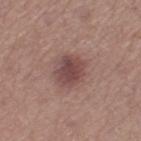biopsy status: catalogued during a skin exam; not biopsied
location: the left thigh
patient: female, in their mid-50s
image-analysis metrics: a lesion area of about 10 mm² and an outline eccentricity of about 0.6 (0 = round, 1 = elongated); a border-irregularity rating of about 2/10 and peripheral color asymmetry of about 1
diameter: about 4 mm
image: ~15 mm crop, total-body skin-cancer survey
tile lighting: white-light illumination